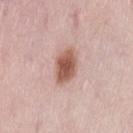{
  "biopsy_status": "not biopsied; imaged during a skin examination",
  "site": "lower back",
  "lesion_size": {
    "long_diameter_mm_approx": 4.0
  },
  "automated_metrics": {
    "eccentricity": 0.75,
    "shape_asymmetry": 0.2,
    "cielab_L": 57,
    "cielab_a": 22,
    "cielab_b": 27,
    "vs_skin_darker_L": 15.0
  },
  "image": {
    "source": "total-body photography crop",
    "field_of_view_mm": 15
  },
  "lighting": "white-light",
  "patient": {
    "sex": "female",
    "age_approx": 50
  }
}